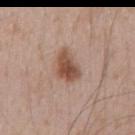The lesion was photographed on a routine skin check and not biopsied; there is no pathology result. On the mid back. Automated tile analysis of the lesion measured a border-irregularity rating of about 3/10, a within-lesion color-variation index near 4/10, and peripheral color asymmetry of about 1. It also reported a lesion-detection confidence of about 100/100. Approximately 4.5 mm at its widest. A male subject aged around 50. A roughly 15 mm field-of-view crop from a total-body skin photograph. This is a white-light tile.